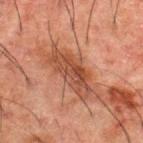Findings:
* biopsy status: no biopsy performed (imaged during a skin exam)
* site: the upper back
* acquisition: ~15 mm crop, total-body skin-cancer survey
* tile lighting: cross-polarized
* patient: male, aged 48 to 52
* automated lesion analysis: an area of roughly 14 mm² and a shape eccentricity near 0.9; a classifier nevus-likeness of about 10/100